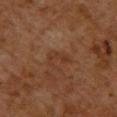Assessment: Recorded during total-body skin imaging; not selected for excision or biopsy. Acquisition and patient details: A male subject approximately 60 years of age. Captured under cross-polarized illumination. A 15 mm crop from a total-body photograph taken for skin-cancer surveillance. Located on the chest.